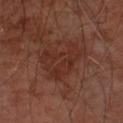biopsy status=imaged on a skin check; not biopsied | diameter=≈5.5 mm | body site=the arm | image source=~15 mm tile from a whole-body skin photo | subject=male, about 65 years old | lighting=cross-polarized.